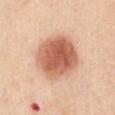biopsy status=catalogued during a skin exam; not biopsied
subject=female, aged around 30
lesion size=≈5.5 mm
imaging modality=15 mm crop, total-body photography
tile lighting=white-light illumination
site=the chest